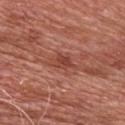Notes:
* notes — no biopsy performed (imaged during a skin exam)